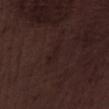Assessment: Recorded during total-body skin imaging; not selected for excision or biopsy. Image and clinical context: The lesion is on the leg. Captured under white-light illumination. The patient is a male about 70 years old. This image is a 15 mm lesion crop taken from a total-body photograph.